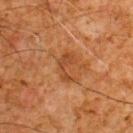<record>
<biopsy_status>not biopsied; imaged during a skin examination</biopsy_status>
</record>